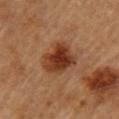Part of a total-body skin-imaging series; this lesion was reviewed on a skin check and was not flagged for biopsy. Automated image analysis of the tile measured an eccentricity of roughly 0.55 and a symmetry-axis asymmetry near 0.2. The analysis additionally found a border-irregularity rating of about 2.5/10, a within-lesion color-variation index near 4.5/10, and a peripheral color-asymmetry measure near 1. And it measured a nevus-likeness score of about 95/100. Captured under cross-polarized illumination. A 15 mm close-up tile from a total-body photography series done for melanoma screening. The subject is a female roughly 60 years of age. On the upper back.